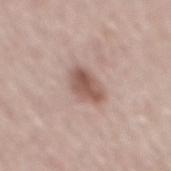Assessment: Imaged during a routine full-body skin examination; the lesion was not biopsied and no histopathology is available. Clinical summary: Automated tile analysis of the lesion measured an area of roughly 7 mm² and a shape-asymmetry score of about 0.2 (0 = symmetric). It also reported an average lesion color of about L≈54 a*≈19 b*≈24 (CIELAB), a lesion–skin lightness drop of about 13, and a normalized lesion–skin contrast near 8.5. It also reported a border-irregularity rating of about 2/10 and a within-lesion color-variation index near 4/10. The lesion is located on the mid back. A male patient aged approximately 65. A roughly 15 mm field-of-view crop from a total-body skin photograph.